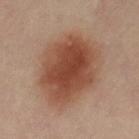Imaged during a routine full-body skin examination; the lesion was not biopsied and no histopathology is available. The lesion is on the left thigh. A female subject aged approximately 40. Imaged with cross-polarized lighting. Approximately 9 mm at its widest. A lesion tile, about 15 mm wide, cut from a 3D total-body photograph.